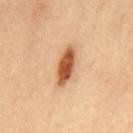The lesion was tiled from a total-body skin photograph and was not biopsied. A region of skin cropped from a whole-body photographic capture, roughly 15 mm wide. Approximately 5 mm at its widest. Captured under cross-polarized illumination. A male subject, aged around 65. The lesion is on the chest.